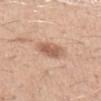The lesion was photographed on a routine skin check and not biopsied; there is no pathology result. Located on the left upper arm. The patient is a male aged 28 to 32. Automated image analysis of the tile measured border irregularity of about 1.5 on a 0–10 scale, internal color variation of about 3 on a 0–10 scale, and radial color variation of about 1. A 15 mm close-up extracted from a 3D total-body photography capture. This is a white-light tile.